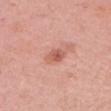No biopsy was performed on this lesion — it was imaged during a full skin examination and was not determined to be concerning. An algorithmic analysis of the crop reported an area of roughly 4 mm² and a shape-asymmetry score of about 0.2 (0 = symmetric). It also reported a border-irregularity rating of about 2.5/10 and a within-lesion color-variation index near 3.5/10. And it measured a classifier nevus-likeness of about 75/100 and lesion-presence confidence of about 100/100. Captured under white-light illumination. The lesion's longest dimension is about 3 mm. From the head or neck. The patient is a female roughly 60 years of age. A roughly 15 mm field-of-view crop from a total-body skin photograph.